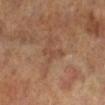Captured during whole-body skin photography for melanoma surveillance; the lesion was not biopsied.